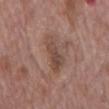Recorded during total-body skin imaging; not selected for excision or biopsy.
A male subject, aged approximately 70.
On the mid back.
Captured under white-light illumination.
The lesion-visualizer software estimated border irregularity of about 4 on a 0–10 scale and a peripheral color-asymmetry measure near 1.5. And it measured an automated nevus-likeness rating near 0 out of 100 and a detector confidence of about 95 out of 100 that the crop contains a lesion.
The recorded lesion diameter is about 5 mm.
A close-up tile cropped from a whole-body skin photograph, about 15 mm across.